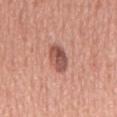Acquisition and patient details: The patient is a male roughly 65 years of age. A roughly 15 mm field-of-view crop from a total-body skin photograph. Located on the front of the torso. The tile uses white-light illumination. Longest diameter approximately 4 mm.